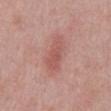Q: Was a biopsy performed?
A: total-body-photography surveillance lesion; no biopsy
Q: Lesion size?
A: about 4.5 mm
Q: Where on the body is the lesion?
A: the front of the torso
Q: What kind of image is this?
A: 15 mm crop, total-body photography
Q: Patient demographics?
A: male, aged approximately 50
Q: What lighting was used for the tile?
A: white-light